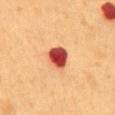- biopsy status: no biopsy performed (imaged during a skin exam)
- lesion size: ≈3 mm
- location: the abdomen
- patient: male, approximately 55 years of age
- acquisition: 15 mm crop, total-body photography